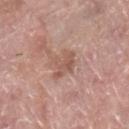No biopsy was performed on this lesion — it was imaged during a full skin examination and was not determined to be concerning.
Imaged with white-light lighting.
A lesion tile, about 15 mm wide, cut from a 3D total-body photograph.
On the right lower leg.
A female subject about 60 years old.